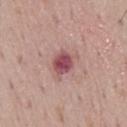| field | value |
|---|---|
| biopsy status | no biopsy performed (imaged during a skin exam) |
| subject | male, approximately 70 years of age |
| image-analysis metrics | about 14 CIELAB-L* units darker than the surrounding skin and a normalized lesion–skin contrast near 10.5; border irregularity of about 2 on a 0–10 scale, a color-variation rating of about 3.5/10, and radial color variation of about 1 |
| illumination | white-light |
| diameter | ~2.5 mm (longest diameter) |
| location | the mid back |
| acquisition | ~15 mm crop, total-body skin-cancer survey |